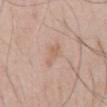{
  "biopsy_status": "not biopsied; imaged during a skin examination"
}